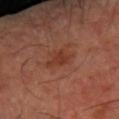Recorded during total-body skin imaging; not selected for excision or biopsy. A 15 mm close-up tile from a total-body photography series done for melanoma screening. Located on the right forearm. A male patient, roughly 65 years of age.